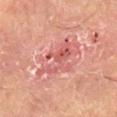This lesion was catalogued during total-body skin photography and was not selected for biopsy. The lesion is located on the right lower leg. A 15 mm crop from a total-body photograph taken for skin-cancer surveillance. A male subject, about 55 years old. Imaged with cross-polarized lighting.